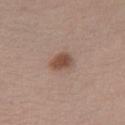lighting = white-light
body site = the chest
lesion size = ≈3 mm
imaging modality = ~15 mm crop, total-body skin-cancer survey
automated lesion analysis = a mean CIELAB color near L≈49 a*≈19 b*≈26 and a normalized lesion–skin contrast near 8.5; border irregularity of about 2 on a 0–10 scale, a color-variation rating of about 3/10, and a peripheral color-asymmetry measure near 1
subject = female, about 25 years old